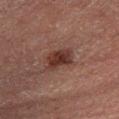workup: catalogued during a skin exam; not biopsied | anatomic site: the left lower leg | imaging modality: ~15 mm tile from a whole-body skin photo | lesion diameter: about 3.5 mm | patient: male, aged around 65 | illumination: cross-polarized.